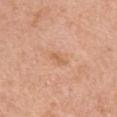Recorded during total-body skin imaging; not selected for excision or biopsy. A female patient, aged approximately 60. From the left upper arm. Captured under white-light illumination. A 15 mm close-up tile from a total-body photography series done for melanoma screening. The total-body-photography lesion software estimated a lesion area of about 2.5 mm², a shape eccentricity near 0.9, and a symmetry-axis asymmetry near 0.35. And it measured a normalized border contrast of about 5.5. It also reported a border-irregularity index near 3.5/10. The software also gave a classifier nevus-likeness of about 0/100 and lesion-presence confidence of about 100/100. Approximately 2.5 mm at its widest.